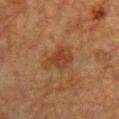Clinical impression: Part of a total-body skin-imaging series; this lesion was reviewed on a skin check and was not flagged for biopsy. Acquisition and patient details: A roughly 15 mm field-of-view crop from a total-body skin photograph. The patient is a female approximately 55 years of age. This is a cross-polarized tile. The lesion is on the front of the torso. Approximately 4 mm at its widest.